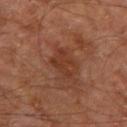Assessment:
Recorded during total-body skin imaging; not selected for excision or biopsy.
Background:
Cropped from a whole-body photographic skin survey; the tile spans about 15 mm. Automated tile analysis of the lesion measured an eccentricity of roughly 0.75 and a symmetry-axis asymmetry near 0.4. It also reported a normalized border contrast of about 6. And it measured a peripheral color-asymmetry measure near 0.5. The lesion's longest dimension is about 3.5 mm. This is a cross-polarized tile. The subject is a male about 60 years old. The lesion is on the right leg.